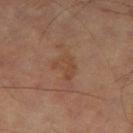<record>
  <biopsy_status>not biopsied; imaged during a skin examination</biopsy_status>
  <image>
    <source>total-body photography crop</source>
    <field_of_view_mm>15</field_of_view_mm>
  </image>
  <automated_metrics>
    <nevus_likeness_0_100>0</nevus_likeness_0_100>
  </automated_metrics>
  <site>left thigh</site>
  <lighting>cross-polarized</lighting>
  <patient>
    <sex>male</sex>
    <age_approx>65</age_approx>
  </patient>
</record>